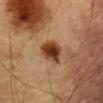{
  "biopsy_status": "not biopsied; imaged during a skin examination",
  "image": {
    "source": "total-body photography crop",
    "field_of_view_mm": 15
  },
  "lesion_size": {
    "long_diameter_mm_approx": 4.0
  },
  "lighting": "cross-polarized",
  "patient": {
    "sex": "male",
    "age_approx": "85+"
  },
  "site": "chest"
}